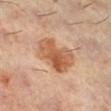Imaged during a routine full-body skin examination; the lesion was not biopsied and no histopathology is available. A 15 mm close-up extracted from a 3D total-body photography capture. Captured under cross-polarized illumination. A female patient aged around 60. From the right lower leg.